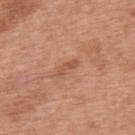The lesion was tiled from a total-body skin photograph and was not biopsied. Longest diameter approximately 3 mm. The lesion-visualizer software estimated a mean CIELAB color near L≈54 a*≈24 b*≈33, a lesion–skin lightness drop of about 8, and a normalized lesion–skin contrast near 5.5. And it measured border irregularity of about 4.5 on a 0–10 scale and radial color variation of about 0. The software also gave a detector confidence of about 100 out of 100 that the crop contains a lesion. Imaged with white-light lighting. A 15 mm close-up tile from a total-body photography series done for melanoma screening. On the upper back. A male patient aged approximately 70.